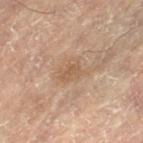<case>
  <biopsy_status>not biopsied; imaged during a skin examination</biopsy_status>
  <automated_metrics>
    <area_mm2_approx>5.0</area_mm2_approx>
    <eccentricity>0.75</eccentricity>
    <shape_asymmetry>0.4</shape_asymmetry>
    <cielab_L>51</cielab_L>
    <cielab_a>16</cielab_a>
    <cielab_b>29</cielab_b>
    <vs_skin_contrast_norm>5.5</vs_skin_contrast_norm>
  </automated_metrics>
  <site>right leg</site>
  <image>
    <source>total-body photography crop</source>
    <field_of_view_mm>15</field_of_view_mm>
  </image>
  <lesion_size>
    <long_diameter_mm_approx>3.0</long_diameter_mm_approx>
  </lesion_size>
  <patient>
    <sex>female</sex>
    <age_approx>80</age_approx>
  </patient>
</case>